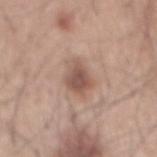notes: total-body-photography surveillance lesion; no biopsy | location: the mid back | lighting: white-light illumination | diameter: ~4 mm (longest diameter) | subject: male, approximately 45 years of age | image: 15 mm crop, total-body photography | image-analysis metrics: a footprint of about 7 mm² and an eccentricity of roughly 0.75; a lesion–skin lightness drop of about 12; a border-irregularity index near 3/10.